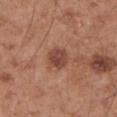The lesion was photographed on a routine skin check and not biopsied; there is no pathology result. A lesion tile, about 15 mm wide, cut from a 3D total-body photograph. Located on the arm. A male patient, roughly 55 years of age.